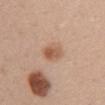patient: female, about 25 years old
tile lighting: white-light illumination
diameter: ~3.5 mm (longest diameter)
imaging modality: ~15 mm crop, total-body skin-cancer survey
body site: the chest